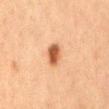Recorded during total-body skin imaging; not selected for excision or biopsy.
The lesion's longest dimension is about 3 mm.
Captured under cross-polarized illumination.
The lesion is located on the mid back.
Cropped from a whole-body photographic skin survey; the tile spans about 15 mm.
A female subject, aged 58 to 62.